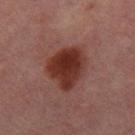{"biopsy_status": "not biopsied; imaged during a skin examination", "lighting": "cross-polarized", "lesion_size": {"long_diameter_mm_approx": 5.0}, "site": "left thigh", "image": {"source": "total-body photography crop", "field_of_view_mm": 15}, "patient": {"sex": "female", "age_approx": 50}}